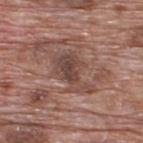follow-up=total-body-photography surveillance lesion; no biopsy
acquisition=~15 mm tile from a whole-body skin photo
subject=male, approximately 70 years of age
anatomic site=the upper back
illumination=white-light illumination
TBP lesion metrics=a lesion color around L≈45 a*≈19 b*≈23 in CIELAB and a lesion–skin lightness drop of about 9; a border-irregularity index near 6/10, a color-variation rating of about 4/10, and radial color variation of about 1.5; a classifier nevus-likeness of about 0/100 and a lesion-detection confidence of about 50/100